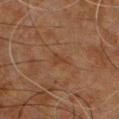The lesion was tiled from a total-body skin photograph and was not biopsied. On the chest. The subject is a male roughly 60 years of age. A region of skin cropped from a whole-body photographic capture, roughly 15 mm wide.